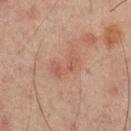Impression: This lesion was catalogued during total-body skin photography and was not selected for biopsy. Context: A close-up tile cropped from a whole-body skin photograph, about 15 mm across. A male patient, in their mid-60s. Automated image analysis of the tile measured a mean CIELAB color near L≈43 a*≈19 b*≈23, about 5 CIELAB-L* units darker than the surrounding skin, and a lesion-to-skin contrast of about 4.5 (normalized; higher = more distinct). The software also gave border irregularity of about 6.5 on a 0–10 scale, a within-lesion color-variation index near 1/10, and peripheral color asymmetry of about 0. Captured under cross-polarized illumination. On the mid back. About 3.5 mm across.